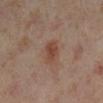No biopsy was performed on this lesion — it was imaged during a full skin examination and was not determined to be concerning.
A female subject, roughly 50 years of age.
About 3.5 mm across.
Located on the left lower leg.
A 15 mm crop from a total-body photograph taken for skin-cancer surveillance.